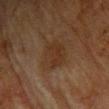- notes: no biopsy performed (imaged during a skin exam)
- location: the chest
- image source: 15 mm crop, total-body photography
- diameter: ~4 mm (longest diameter)
- patient: female, roughly 80 years of age
- illumination: cross-polarized illumination